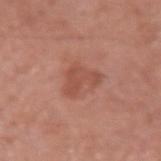notes: no biopsy performed (imaged during a skin exam)
TBP lesion metrics: an average lesion color of about L≈51 a*≈26 b*≈29 (CIELAB), about 8 CIELAB-L* units darker than the surrounding skin, and a normalized border contrast of about 5.5; border irregularity of about 6.5 on a 0–10 scale, internal color variation of about 2 on a 0–10 scale, and peripheral color asymmetry of about 0.5; an automated nevus-likeness rating near 0 out of 100 and lesion-presence confidence of about 100/100
size: ~3.5 mm (longest diameter)
subject: male, about 55 years old
lighting: white-light
image: total-body-photography crop, ~15 mm field of view
site: the left upper arm